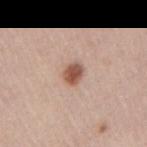Q: Was this lesion biopsied?
A: total-body-photography surveillance lesion; no biopsy
Q: Lesion size?
A: ~2.5 mm (longest diameter)
Q: What is the anatomic site?
A: the right upper arm
Q: Automated lesion metrics?
A: border irregularity of about 2 on a 0–10 scale and radial color variation of about 1; an automated nevus-likeness rating near 95 out of 100 and lesion-presence confidence of about 100/100
Q: What are the patient's age and sex?
A: male, aged approximately 45
Q: How was this image acquired?
A: ~15 mm tile from a whole-body skin photo
Q: What lighting was used for the tile?
A: white-light illumination